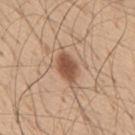Notes:
• notes · total-body-photography surveillance lesion; no biopsy
• site · the mid back
• patient · male, aged approximately 55
• tile lighting · white-light illumination
• imaging modality · total-body-photography crop, ~15 mm field of view